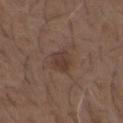Assessment:
Imaged during a routine full-body skin examination; the lesion was not biopsied and no histopathology is available.
Background:
Automated tile analysis of the lesion measured a footprint of about 5.5 mm², an eccentricity of roughly 0.7, and a symmetry-axis asymmetry near 0.25. The analysis additionally found a mean CIELAB color near L≈37 a*≈16 b*≈22, a lesion–skin lightness drop of about 7, and a normalized border contrast of about 6.5. Approximately 3.5 mm at its widest. The subject is a male aged around 50. The lesion is located on the chest. This image is a 15 mm lesion crop taken from a total-body photograph.